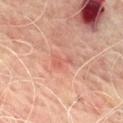Recorded during total-body skin imaging; not selected for excision or biopsy. The lesion is located on the upper back. This is a cross-polarized tile. A region of skin cropped from a whole-body photographic capture, roughly 15 mm wide. About 2.5 mm across. A male subject, aged approximately 70.